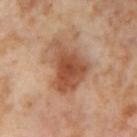* notes · total-body-photography surveillance lesion; no biopsy
* illumination · cross-polarized illumination
* lesion size · about 6 mm
* subject · female, about 55 years old
* body site · the leg
* image source · ~15 mm crop, total-body skin-cancer survey